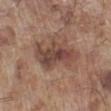Recorded during total-body skin imaging; not selected for excision or biopsy.
Longest diameter approximately 6.5 mm.
A 15 mm crop from a total-body photograph taken for skin-cancer surveillance.
A male patient aged 68 to 72.
The lesion is located on the left lower leg.
Automated tile analysis of the lesion measured an area of roughly 16 mm², an outline eccentricity of about 0.85 (0 = round, 1 = elongated), and two-axis asymmetry of about 0.3. The software also gave an average lesion color of about L≈45 a*≈19 b*≈24 (CIELAB) and a lesion–skin lightness drop of about 10. The analysis additionally found an automated nevus-likeness rating near 5 out of 100.